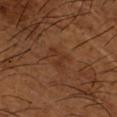Q: How was this image acquired?
A: total-body-photography crop, ~15 mm field of view
Q: Who is the patient?
A: male, about 65 years old
Q: How large is the lesion?
A: about 3.5 mm
Q: What did automated image analysis measure?
A: a color-variation rating of about 1.5/10; a nevus-likeness score of about 0/100
Q: Illumination type?
A: cross-polarized
Q: Lesion location?
A: the left forearm